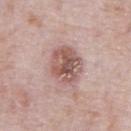The lesion was tiled from a total-body skin photograph and was not biopsied. A male patient roughly 70 years of age. A close-up tile cropped from a whole-body skin photograph, about 15 mm across. The lesion is located on the abdomen.